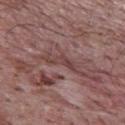Automated tile analysis of the lesion measured a lesion area of about 4 mm², a shape eccentricity near 0.95, and a shape-asymmetry score of about 0.55 (0 = symmetric). The analysis additionally found a mean CIELAB color near L≈42 a*≈21 b*≈20, a lesion–skin lightness drop of about 8, and a normalized border contrast of about 6.5. The software also gave border irregularity of about 7 on a 0–10 scale, internal color variation of about 0 on a 0–10 scale, and a peripheral color-asymmetry measure near 0. The patient is a male aged 68 to 72. The recorded lesion diameter is about 4 mm. Located on the upper back. A 15 mm crop from a total-body photograph taken for skin-cancer surveillance.